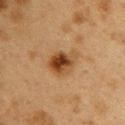{
  "biopsy_status": "not biopsied; imaged during a skin examination",
  "site": "upper back",
  "patient": {
    "sex": "female",
    "age_approx": 40
  },
  "automated_metrics": {
    "cielab_L": 38,
    "cielab_a": 19,
    "cielab_b": 33,
    "vs_skin_darker_L": 11.0,
    "vs_skin_contrast_norm": 10.0,
    "border_irregularity_0_10": 2.5,
    "color_variation_0_10": 8.5
  },
  "image": {
    "source": "total-body photography crop",
    "field_of_view_mm": 15
  }
}